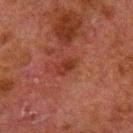{
  "biopsy_status": "not biopsied; imaged during a skin examination",
  "site": "leg",
  "image": {
    "source": "total-body photography crop",
    "field_of_view_mm": 15
  },
  "lighting": "cross-polarized",
  "lesion_size": {
    "long_diameter_mm_approx": 2.5
  },
  "patient": {
    "sex": "male",
    "age_approx": 80
  }
}